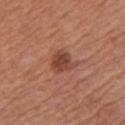{"patient": {"sex": "female", "age_approx": 65}, "site": "left upper arm", "image": {"source": "total-body photography crop", "field_of_view_mm": 15}}